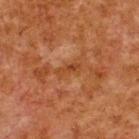– follow-up · catalogued during a skin exam; not biopsied
– illumination · cross-polarized illumination
– body site · the upper back
– acquisition · 15 mm crop, total-body photography
– diameter · ≈3 mm
– patient · male, aged 78–82